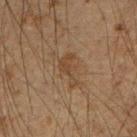No biopsy was performed on this lesion — it was imaged during a full skin examination and was not determined to be concerning. From the right upper arm. The tile uses cross-polarized illumination. The subject is a male approximately 50 years of age. Cropped from a total-body skin-imaging series; the visible field is about 15 mm. The lesion's longest dimension is about 4 mm.